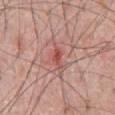Part of a total-body skin-imaging series; this lesion was reviewed on a skin check and was not flagged for biopsy.